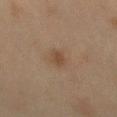| feature | finding |
|---|---|
| workup | imaged on a skin check; not biopsied |
| tile lighting | cross-polarized illumination |
| patient | male, aged 43 to 47 |
| site | the mid back |
| imaging modality | total-body-photography crop, ~15 mm field of view |
| diameter | ≈2.5 mm |